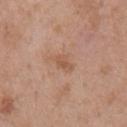Assessment:
Part of a total-body skin-imaging series; this lesion was reviewed on a skin check and was not flagged for biopsy.
Acquisition and patient details:
A male subject aged 53 to 57. Located on the chest. A 15 mm close-up extracted from a 3D total-body photography capture.